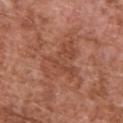Q: Where on the body is the lesion?
A: the upper back
Q: What is the imaging modality?
A: ~15 mm tile from a whole-body skin photo
Q: What are the patient's age and sex?
A: male, aged around 75
Q: Illumination type?
A: white-light